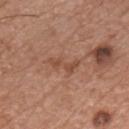Captured during whole-body skin photography for melanoma surveillance; the lesion was not biopsied.
Longest diameter approximately 3.5 mm.
A male patient in their mid- to late 70s.
The lesion is on the chest.
Automated tile analysis of the lesion measured a color-variation rating of about 0/10 and a peripheral color-asymmetry measure near 0.
Imaged with white-light lighting.
A region of skin cropped from a whole-body photographic capture, roughly 15 mm wide.